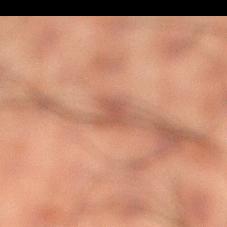* follow-up — imaged on a skin check; not biopsied
* subject — male, aged around 50
* acquisition — ~15 mm tile from a whole-body skin photo
* body site — the leg
* TBP lesion metrics — a lesion color around L≈41 a*≈19 b*≈25 in CIELAB, roughly 8 lightness units darker than nearby skin, and a lesion-to-skin contrast of about 6.5 (normalized; higher = more distinct); a border-irregularity rating of about 4.5/10, a within-lesion color-variation index near 1/10, and radial color variation of about 0.5; a classifier nevus-likeness of about 0/100 and lesion-presence confidence of about 90/100
* illumination — cross-polarized illumination
* size — about 2.5 mm